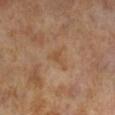Recorded during total-body skin imaging; not selected for excision or biopsy. A male subject, in their mid-60s. Automated tile analysis of the lesion measured an area of roughly 4 mm², a shape eccentricity near 0.6, and two-axis asymmetry of about 0.55. The software also gave border irregularity of about 6.5 on a 0–10 scale. A roughly 15 mm field-of-view crop from a total-body skin photograph. The lesion's longest dimension is about 3 mm. This is a cross-polarized tile.